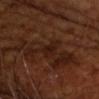Impression:
This lesion was catalogued during total-body skin photography and was not selected for biopsy.
Acquisition and patient details:
The patient is a male approximately 65 years of age. Cropped from a total-body skin-imaging series; the visible field is about 15 mm.